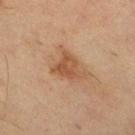Captured during whole-body skin photography for melanoma surveillance; the lesion was not biopsied. The tile uses cross-polarized illumination. The lesion's longest dimension is about 4 mm. A female subject, about 55 years old. A 15 mm crop from a total-body photograph taken for skin-cancer surveillance. Located on the right lower leg. The total-body-photography lesion software estimated a lesion area of about 7 mm², an outline eccentricity of about 0.55 (0 = round, 1 = elongated), and a shape-asymmetry score of about 0.45 (0 = symmetric). It also reported a lesion–skin lightness drop of about 9 and a lesion-to-skin contrast of about 7 (normalized; higher = more distinct). The software also gave a border-irregularity rating of about 5/10, a color-variation rating of about 3.5/10, and radial color variation of about 1. It also reported lesion-presence confidence of about 100/100.